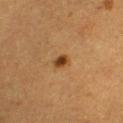Clinical impression: No biopsy was performed on this lesion — it was imaged during a full skin examination and was not determined to be concerning. Context: A 15 mm crop from a total-body photograph taken for skin-cancer surveillance. A female subject, approximately 30 years of age. The tile uses cross-polarized illumination. The lesion's longest dimension is about 2 mm. Located on the chest. Automated image analysis of the tile measured about 12 CIELAB-L* units darker than the surrounding skin and a lesion-to-skin contrast of about 9.5 (normalized; higher = more distinct). The software also gave internal color variation of about 4 on a 0–10 scale and radial color variation of about 1.5. It also reported an automated nevus-likeness rating near 100 out of 100 and a lesion-detection confidence of about 100/100.